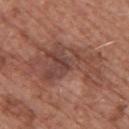patient: male, aged 73 to 77
image: ~15 mm crop, total-body skin-cancer survey
body site: the upper back